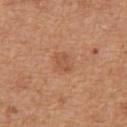A close-up tile cropped from a whole-body skin photograph, about 15 mm across. The lesion is located on the front of the torso. A male subject, approximately 65 years of age. Captured under white-light illumination.